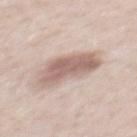This lesion was catalogued during total-body skin photography and was not selected for biopsy.
A region of skin cropped from a whole-body photographic capture, roughly 15 mm wide.
The lesion is on the back.
The subject is a male about 40 years old.
This is a white-light tile.
Longest diameter approximately 6 mm.